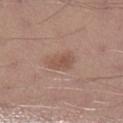Impression: The lesion was tiled from a total-body skin photograph and was not biopsied. Acquisition and patient details: The lesion is on the left lower leg. A male patient, aged 28–32. Cropped from a total-body skin-imaging series; the visible field is about 15 mm.